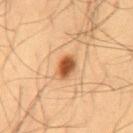The lesion was photographed on a routine skin check and not biopsied; there is no pathology result.
A male patient, aged 53 to 57.
About 3 mm across.
This is a cross-polarized tile.
The lesion is located on the mid back.
A 15 mm close-up extracted from a 3D total-body photography capture.